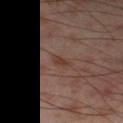{
  "biopsy_status": "not biopsied; imaged during a skin examination",
  "site": "left thigh",
  "image": {
    "source": "total-body photography crop",
    "field_of_view_mm": 15
  },
  "patient": {
    "sex": "male",
    "age_approx": 55
  },
  "lesion_size": {
    "long_diameter_mm_approx": 2.5
  }
}